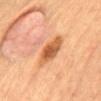The lesion was photographed on a routine skin check and not biopsied; there is no pathology result.
Approximately 5 mm at its widest.
The lesion is located on the abdomen.
A roughly 15 mm field-of-view crop from a total-body skin photograph.
A male subject, aged 58 to 62.
The lesion-visualizer software estimated an eccentricity of roughly 0.85 and a symmetry-axis asymmetry near 0.15. The analysis additionally found roughly 13 lightness units darker than nearby skin and a lesion-to-skin contrast of about 9 (normalized; higher = more distinct). It also reported a border-irregularity index near 2/10, internal color variation of about 4.5 on a 0–10 scale, and peripheral color asymmetry of about 1.